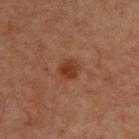Captured during whole-body skin photography for melanoma surveillance; the lesion was not biopsied.
A region of skin cropped from a whole-body photographic capture, roughly 15 mm wide.
The lesion is on the upper back.
The recorded lesion diameter is about 2.5 mm.
The lesion-visualizer software estimated a footprint of about 5 mm², an eccentricity of roughly 0.4, and a shape-asymmetry score of about 0.2 (0 = symmetric).
A male patient, approximately 60 years of age.